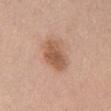No biopsy was performed on this lesion — it was imaged during a full skin examination and was not determined to be concerning. Imaged with white-light lighting. The lesion's longest dimension is about 4.5 mm. The lesion is on the front of the torso. This image is a 15 mm lesion crop taken from a total-body photograph. A male subject aged 53–57.